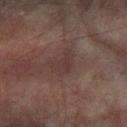Notes:
* notes — no biopsy performed (imaged during a skin exam)
* anatomic site — the left forearm
* illumination — cross-polarized illumination
* subject — male, aged 73–77
* acquisition — total-body-photography crop, ~15 mm field of view
* lesion size — about 3 mm